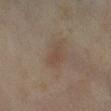{"biopsy_status": "not biopsied; imaged during a skin examination", "lesion_size": {"long_diameter_mm_approx": 2.5}, "site": "left lower leg", "image": {"source": "total-body photography crop", "field_of_view_mm": 15}, "lighting": "cross-polarized", "patient": {"sex": "female", "age_approx": 60}}